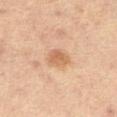Assessment: Recorded during total-body skin imaging; not selected for excision or biopsy. Context: The total-body-photography lesion software estimated a lesion area of about 5.5 mm², an outline eccentricity of about 0.4 (0 = round, 1 = elongated), and two-axis asymmetry of about 0.2. And it measured a lesion color around L≈61 a*≈21 b*≈35 in CIELAB. The analysis additionally found border irregularity of about 2 on a 0–10 scale, a within-lesion color-variation index near 3/10, and radial color variation of about 1. A lesion tile, about 15 mm wide, cut from a 3D total-body photograph. Measured at roughly 2.5 mm in maximum diameter. The lesion is on the left thigh. The patient is a male roughly 55 years of age.